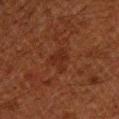follow-up: imaged on a skin check; not biopsied
location: the left upper arm
imaging modality: ~15 mm tile from a whole-body skin photo
tile lighting: cross-polarized
patient: male, in their 60s